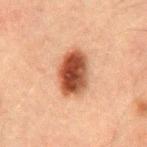Clinical impression:
Recorded during total-body skin imaging; not selected for excision or biopsy.
Acquisition and patient details:
A male patient, aged 48–52. This is a cross-polarized tile. A lesion tile, about 15 mm wide, cut from a 3D total-body photograph. The lesion-visualizer software estimated a footprint of about 13 mm², an outline eccentricity of about 0.75 (0 = round, 1 = elongated), and a shape-asymmetry score of about 0.15 (0 = symmetric). It also reported border irregularity of about 1.5 on a 0–10 scale, a within-lesion color-variation index near 7/10, and radial color variation of about 2. The analysis additionally found a nevus-likeness score of about 100/100 and a lesion-detection confidence of about 100/100. The lesion is on the mid back. Measured at roughly 5 mm in maximum diameter.